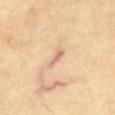Q: Was this lesion biopsied?
A: no biopsy performed (imaged during a skin exam)
Q: What is the imaging modality?
A: 15 mm crop, total-body photography
Q: Patient demographics?
A: male, in their 70s
Q: What is the lesion's diameter?
A: ≈2.5 mm
Q: Illumination type?
A: cross-polarized illumination
Q: Lesion location?
A: the chest